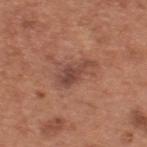biopsy_status: not biopsied; imaged during a skin examination
lighting: white-light
patient:
  sex: male
  age_approx: 65
lesion_size:
  long_diameter_mm_approx: 4.0
image:
  source: total-body photography crop
  field_of_view_mm: 15
site: upper back